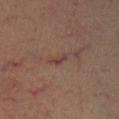Clinical impression: This lesion was catalogued during total-body skin photography and was not selected for biopsy. Acquisition and patient details: The tile uses cross-polarized illumination. A male subject, about 60 years old. Located on the left lower leg. A 15 mm close-up extracted from a 3D total-body photography capture. An algorithmic analysis of the crop reported a nevus-likeness score of about 0/100. Approximately 2.5 mm at its widest.